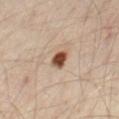Part of a total-body skin-imaging series; this lesion was reviewed on a skin check and was not flagged for biopsy.